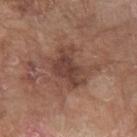Image and clinical context:
A female subject, aged approximately 75. A region of skin cropped from a whole-body photographic capture, roughly 15 mm wide. The lesion is located on the right forearm. Approximately 5 mm at its widest. Automated tile analysis of the lesion measured a footprint of about 13 mm², an outline eccentricity of about 0.75 (0 = round, 1 = elongated), and a symmetry-axis asymmetry near 0.35. The software also gave roughly 9 lightness units darker than nearby skin and a normalized border contrast of about 7.5. The analysis additionally found a border-irregularity index near 4/10 and peripheral color asymmetry of about 1.5. It also reported a classifier nevus-likeness of about 15/100 and a lesion-detection confidence of about 100/100. The tile uses white-light illumination.